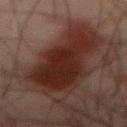<tbp_lesion>
  <biopsy_status>not biopsied; imaged during a skin examination</biopsy_status>
  <image>
    <source>total-body photography crop</source>
    <field_of_view_mm>15</field_of_view_mm>
  </image>
  <site>back</site>
  <lighting>cross-polarized</lighting>
  <patient>
    <sex>male</sex>
    <age_approx>70</age_approx>
  </patient>
</tbp_lesion>